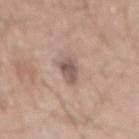Impression: No biopsy was performed on this lesion — it was imaged during a full skin examination and was not determined to be concerning.